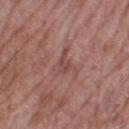body site = the left thigh | subject = female, in their 70s | automated metrics = an average lesion color of about L≈47 a*≈22 b*≈23 (CIELAB), about 7 CIELAB-L* units darker than the surrounding skin, and a normalized border contrast of about 5.5; a within-lesion color-variation index near 3/10 and radial color variation of about 1; a lesion-detection confidence of about 80/100 | acquisition = total-body-photography crop, ~15 mm field of view | size = about 3.5 mm | tile lighting = white-light illumination.